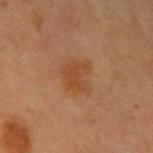This lesion was catalogued during total-body skin photography and was not selected for biopsy.
Imaged with cross-polarized lighting.
A male subject, aged 63–67.
A region of skin cropped from a whole-body photographic capture, roughly 15 mm wide.
Automated tile analysis of the lesion measured a lesion area of about 11 mm², an eccentricity of roughly 0.55, and two-axis asymmetry of about 0.25. The analysis additionally found an average lesion color of about L≈37 a*≈18 b*≈29 (CIELAB) and about 5 CIELAB-L* units darker than the surrounding skin. And it measured a nevus-likeness score of about 40/100 and a lesion-detection confidence of about 100/100.
From the arm.
Measured at roughly 4 mm in maximum diameter.